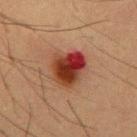Captured during whole-body skin photography for melanoma surveillance; the lesion was not biopsied. Measured at roughly 4.5 mm in maximum diameter. Automated tile analysis of the lesion measured an outline eccentricity of about 0.55 (0 = round, 1 = elongated). The software also gave radial color variation of about 5.5. Cropped from a whole-body photographic skin survey; the tile spans about 15 mm. On the chest. A male subject, about 55 years old. The tile uses cross-polarized illumination.